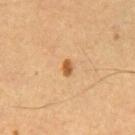Assessment:
The lesion was photographed on a routine skin check and not biopsied; there is no pathology result.
Clinical summary:
Captured under cross-polarized illumination. Approximately 2 mm at its widest. The patient is a male aged around 55. Automated tile analysis of the lesion measured a classifier nevus-likeness of about 95/100 and lesion-presence confidence of about 100/100. On the front of the torso. Cropped from a total-body skin-imaging series; the visible field is about 15 mm.